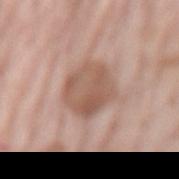Findings:
- workup — no biopsy performed (imaged during a skin exam)
- imaging modality — total-body-photography crop, ~15 mm field of view
- body site — the mid back
- patient — female, in their mid- to late 70s
- lighting — white-light
- size — ≈5 mm